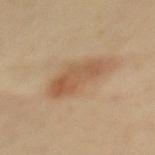biopsy status: catalogued during a skin exam; not biopsied | lighting: cross-polarized | subject: female, aged 38–42 | imaging modality: total-body-photography crop, ~15 mm field of view | body site: the mid back | lesion size: ≈6 mm.